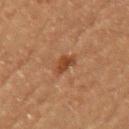Captured during whole-body skin photography for melanoma surveillance; the lesion was not biopsied.